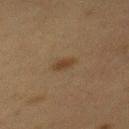Imaged during a routine full-body skin examination; the lesion was not biopsied and no histopathology is available.
The lesion is on the mid back.
A lesion tile, about 15 mm wide, cut from a 3D total-body photograph.
A female patient, aged around 40.
This is a cross-polarized tile.